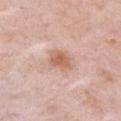{"biopsy_status": "not biopsied; imaged during a skin examination", "image": {"source": "total-body photography crop", "field_of_view_mm": 15}, "site": "chest", "lighting": "white-light", "automated_metrics": {"area_mm2_approx": 6.0, "eccentricity": 0.65, "shape_asymmetry": 0.2, "color_variation_0_10": 2.5, "peripheral_color_asymmetry": 0.5, "lesion_detection_confidence_0_100": 100}, "patient": {"sex": "female", "age_approx": 60}}